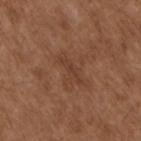Case summary:
– follow-up: imaged on a skin check; not biopsied
– site: the left upper arm
– image source: ~15 mm tile from a whole-body skin photo
– tile lighting: white-light
– patient: male, aged 73 to 77